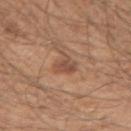follow-up: catalogued during a skin exam; not biopsied | location: the right upper arm | image source: 15 mm crop, total-body photography | subject: male, in their 50s.